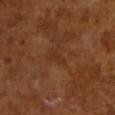Q: How was this image acquired?
A: total-body-photography crop, ~15 mm field of view
Q: Lesion size?
A: about 2.5 mm
Q: Patient demographics?
A: male, aged 63 to 67
Q: How was the tile lit?
A: cross-polarized